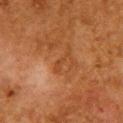workup=total-body-photography surveillance lesion; no biopsy
patient=female, aged around 50
body site=the chest
diameter=~3 mm (longest diameter)
image=~15 mm tile from a whole-body skin photo
automated lesion analysis=an area of roughly 2.5 mm², an eccentricity of roughly 0.95, and a symmetry-axis asymmetry near 0.5
tile lighting=cross-polarized